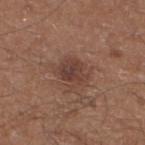Captured during whole-body skin photography for melanoma surveillance; the lesion was not biopsied. The tile uses white-light illumination. The lesion is located on the left lower leg. The patient is a male approximately 50 years of age. A region of skin cropped from a whole-body photographic capture, roughly 15 mm wide. The recorded lesion diameter is about 3.5 mm.